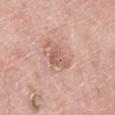Assessment: Part of a total-body skin-imaging series; this lesion was reviewed on a skin check and was not flagged for biopsy. Clinical summary: A male subject, approximately 55 years of age. On the right lower leg. Cropped from a total-body skin-imaging series; the visible field is about 15 mm. About 2.5 mm across.